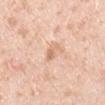biopsy_status: not biopsied; imaged during a skin examination
site: left upper arm
lesion_size:
  long_diameter_mm_approx: 2.5
image:
  source: total-body photography crop
  field_of_view_mm: 15
automated_metrics:
  border_irregularity_0_10: 3.5
  color_variation_0_10: 1.0
  peripheral_color_asymmetry: 0.5
  nevus_likeness_0_100: 0
  lesion_detection_confidence_0_100: 100
patient:
  sex: male
  age_approx: 40